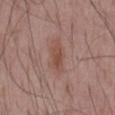{"biopsy_status": "not biopsied; imaged during a skin examination", "lighting": "white-light", "image": {"source": "total-body photography crop", "field_of_view_mm": 15}, "site": "mid back", "lesion_size": {"long_diameter_mm_approx": 3.5}, "patient": {"sex": "male", "age_approx": 55}}